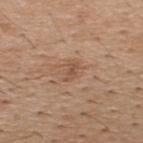Imaged during a routine full-body skin examination; the lesion was not biopsied and no histopathology is available. A lesion tile, about 15 mm wide, cut from a 3D total-body photograph. Located on the back. A male subject, aged approximately 40.